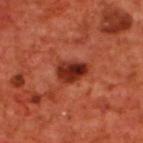biopsy status: no biopsy performed (imaged during a skin exam)
image-analysis metrics: an area of roughly 7 mm², a shape eccentricity near 0.75, and a symmetry-axis asymmetry near 0.25; about 14 CIELAB-L* units darker than the surrounding skin; a border-irregularity index near 2/10, a within-lesion color-variation index near 7.5/10, and peripheral color asymmetry of about 2.5
patient: male, roughly 70 years of age
image: 15 mm crop, total-body photography
anatomic site: the upper back
illumination: cross-polarized illumination
lesion size: ~3.5 mm (longest diameter)